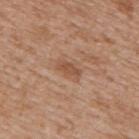Captured during whole-body skin photography for melanoma surveillance; the lesion was not biopsied. Automated tile analysis of the lesion measured border irregularity of about 2 on a 0–10 scale and radial color variation of about 0.5. The analysis additionally found a lesion-detection confidence of about 100/100. The subject is a male aged 63 to 67. Imaged with white-light lighting. From the mid back. This image is a 15 mm lesion crop taken from a total-body photograph.